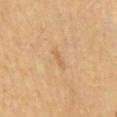{
  "biopsy_status": "not biopsied; imaged during a skin examination",
  "site": "mid back",
  "patient": {
    "sex": "female",
    "age_approx": 60
  },
  "lighting": "cross-polarized",
  "automated_metrics": {
    "cielab_L": 63,
    "cielab_a": 20,
    "cielab_b": 41,
    "vs_skin_contrast_norm": 5.5,
    "nevus_likeness_0_100": 0,
    "lesion_detection_confidence_0_100": 100
  },
  "image": {
    "source": "total-body photography crop",
    "field_of_view_mm": 15
  }
}